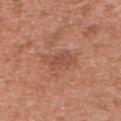Imaged during a routine full-body skin examination; the lesion was not biopsied and no histopathology is available.
A roughly 15 mm field-of-view crop from a total-body skin photograph.
A female patient, aged approximately 35.
The lesion is on the upper back.